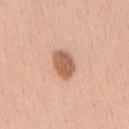Clinical impression:
Part of a total-body skin-imaging series; this lesion was reviewed on a skin check and was not flagged for biopsy.
Context:
On the mid back. An algorithmic analysis of the crop reported roughly 15 lightness units darker than nearby skin and a lesion-to-skin contrast of about 9 (normalized; higher = more distinct). The analysis additionally found border irregularity of about 1.5 on a 0–10 scale, internal color variation of about 2 on a 0–10 scale, and a peripheral color-asymmetry measure near 0.5. The analysis additionally found an automated nevus-likeness rating near 95 out of 100 and lesion-presence confidence of about 100/100. A 15 mm close-up extracted from a 3D total-body photography capture. The subject is a male about 40 years old. The lesion's longest dimension is about 3.5 mm.